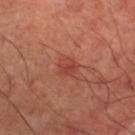{
  "image": {
    "source": "total-body photography crop",
    "field_of_view_mm": 15
  },
  "patient": {
    "sex": "male",
    "age_approx": 65
  },
  "site": "right lower leg",
  "lighting": "cross-polarized",
  "automated_metrics": {
    "area_mm2_approx": 4.0,
    "cielab_L": 44,
    "cielab_a": 31,
    "cielab_b": 30,
    "vs_skin_darker_L": 7.0,
    "vs_skin_contrast_norm": 5.5,
    "border_irregularity_0_10": 2.5,
    "color_variation_0_10": 2.5,
    "peripheral_color_asymmetry": 1.0
  },
  "lesion_size": {
    "long_diameter_mm_approx": 2.5
  }
}